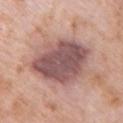<record>
  <biopsy_status>not biopsied; imaged during a skin examination</biopsy_status>
  <lesion_size>
    <long_diameter_mm_approx>7.5</long_diameter_mm_approx>
  </lesion_size>
  <image>
    <source>total-body photography crop</source>
    <field_of_view_mm>15</field_of_view_mm>
  </image>
  <lighting>white-light</lighting>
  <patient>
    <sex>female</sex>
    <age_approx>70</age_approx>
  </patient>
  <site>right upper arm</site>
</record>